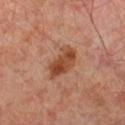{
  "lighting": "cross-polarized",
  "lesion_size": {
    "long_diameter_mm_approx": 4.5
  },
  "image": {
    "source": "total-body photography crop",
    "field_of_view_mm": 15
  },
  "site": "left leg",
  "patient": {
    "sex": "male",
    "age_approx": 50
  }
}